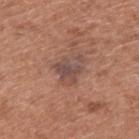biopsy_status: not biopsied; imaged during a skin examination
site: back
lesion_size:
  long_diameter_mm_approx: 4.0
lighting: white-light
image:
  source: total-body photography crop
  field_of_view_mm: 15
patient:
  sex: male
  age_approx: 55